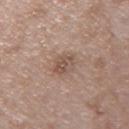The lesion was tiled from a total-body skin photograph and was not biopsied. Measured at roughly 3 mm in maximum diameter. A 15 mm close-up extracted from a 3D total-body photography capture. A male subject aged 48–52. The lesion is on the arm. The tile uses white-light illumination.